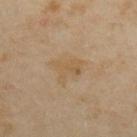Recorded during total-body skin imaging; not selected for excision or biopsy.
A female patient aged approximately 35.
From the upper back.
Cropped from a total-body skin-imaging series; the visible field is about 15 mm.
Captured under cross-polarized illumination.
The lesion's longest dimension is about 4 mm.